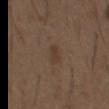Captured during whole-body skin photography for melanoma surveillance; the lesion was not biopsied. A lesion tile, about 15 mm wide, cut from a 3D total-body photograph. About 2.5 mm across. The patient is a male in their 50s. From the chest. The tile uses white-light illumination. The lesion-visualizer software estimated a footprint of about 2.5 mm². The software also gave an average lesion color of about L≈37 a*≈14 b*≈25 (CIELAB) and a normalized lesion–skin contrast near 5.5. And it measured a nevus-likeness score of about 5/100 and lesion-presence confidence of about 100/100.